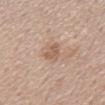biopsy_status: not biopsied; imaged during a skin examination
patient:
  sex: male
  age_approx: 60
lighting: white-light
image:
  source: total-body photography crop
  field_of_view_mm: 15
site: mid back